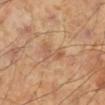The lesion was tiled from a total-body skin photograph and was not biopsied.